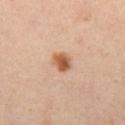| field | value |
|---|---|
| location | the left thigh |
| lesion size | about 3 mm |
| image source | ~15 mm tile from a whole-body skin photo |
| subject | female, aged approximately 40 |
| lighting | cross-polarized |